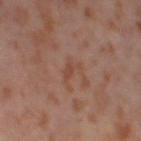Image and clinical context:
Measured at roughly 2.5 mm in maximum diameter. A female subject, in their mid- to late 50s. A lesion tile, about 15 mm wide, cut from a 3D total-body photograph. The lesion is located on the left thigh.